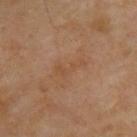The lesion is on the upper back. A male subject, aged 68–72. Cropped from a total-body skin-imaging series; the visible field is about 15 mm.